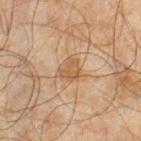notes = imaged on a skin check; not biopsied | size = ≈3 mm | body site = the right lower leg | imaging modality = ~15 mm crop, total-body skin-cancer survey | patient = male, aged 43–47 | TBP lesion metrics = an average lesion color of about L≈46 a*≈15 b*≈30 (CIELAB) and a normalized lesion–skin contrast near 6.5; an automated nevus-likeness rating near 5 out of 100.